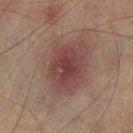Captured during whole-body skin photography for melanoma surveillance; the lesion was not biopsied. Cropped from a whole-body photographic skin survey; the tile spans about 15 mm. The lesion is located on the left lower leg. The tile uses cross-polarized illumination. The patient is a male in their mid-70s.